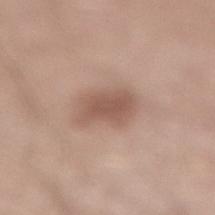| key | value |
|---|---|
| follow-up | catalogued during a skin exam; not biopsied |
| size | ≈4.5 mm |
| subject | male, in their 30s |
| illumination | white-light |
| site | the leg |
| automated lesion analysis | a mean CIELAB color near L≈55 a*≈19 b*≈26, about 11 CIELAB-L* units darker than the surrounding skin, and a normalized lesion–skin contrast near 7; a border-irregularity rating of about 2.5/10, a within-lesion color-variation index near 2.5/10, and peripheral color asymmetry of about 0.5; a nevus-likeness score of about 70/100 and a lesion-detection confidence of about 100/100 |
| image | ~15 mm tile from a whole-body skin photo |